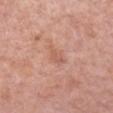Impression:
This lesion was catalogued during total-body skin photography and was not selected for biopsy.
Clinical summary:
Automated tile analysis of the lesion measured a nevus-likeness score of about 0/100 and a lesion-detection confidence of about 100/100. About 3 mm across. Imaged with white-light lighting. On the arm. A 15 mm crop from a total-body photograph taken for skin-cancer surveillance. The subject is a female approximately 60 years of age.